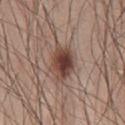Imaged during a routine full-body skin examination; the lesion was not biopsied and no histopathology is available. The subject is a male about 45 years old. A region of skin cropped from a whole-body photographic capture, roughly 15 mm wide. Automated image analysis of the tile measured a footprint of about 12 mm², a shape eccentricity near 0.75, and a shape-asymmetry score of about 0.25 (0 = symmetric). And it measured peripheral color asymmetry of about 2. And it measured an automated nevus-likeness rating near 100 out of 100 and a detector confidence of about 100 out of 100 that the crop contains a lesion. Imaged with white-light lighting. From the chest. Longest diameter approximately 5 mm.